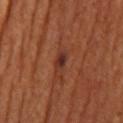{"biopsy_status": "not biopsied; imaged during a skin examination"}